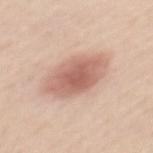Image and clinical context: A 15 mm crop from a total-body photograph taken for skin-cancer surveillance. On the back. The subject is a female aged approximately 50.